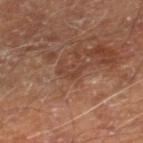A 15 mm close-up extracted from a 3D total-body photography capture. Approximately 3 mm at its widest. A male subject, aged 68 to 72. From the leg.